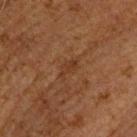This lesion was catalogued during total-body skin photography and was not selected for biopsy. The subject is a male aged approximately 65. The lesion is located on the head or neck. A roughly 15 mm field-of-view crop from a total-body skin photograph.